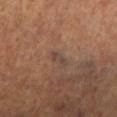No biopsy was performed on this lesion — it was imaged during a full skin examination and was not determined to be concerning.
The lesion-visualizer software estimated an average lesion color of about L≈39 a*≈16 b*≈22 (CIELAB) and about 6 CIELAB-L* units darker than the surrounding skin.
Longest diameter approximately 2.5 mm.
A female subject, aged approximately 65.
A region of skin cropped from a whole-body photographic capture, roughly 15 mm wide.
On the left lower leg.